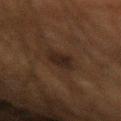Acquisition and patient details: A male patient, aged around 60. The recorded lesion diameter is about 3.5 mm. The tile uses cross-polarized illumination. On the left forearm. Automated tile analysis of the lesion measured a footprint of about 7 mm², an eccentricity of roughly 0.55, and a shape-asymmetry score of about 0.2 (0 = symmetric). And it measured a nevus-likeness score of about 0/100 and a lesion-detection confidence of about 100/100. Cropped from a whole-body photographic skin survey; the tile spans about 15 mm.